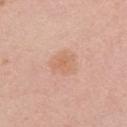Q: Lesion size?
A: ~3.5 mm (longest diameter)
Q: How was this image acquired?
A: 15 mm crop, total-body photography
Q: What are the patient's age and sex?
A: female, roughly 25 years of age
Q: What is the anatomic site?
A: the right upper arm
Q: What lighting was used for the tile?
A: white-light illumination